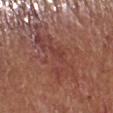Case summary:
* notes: no biopsy performed (imaged during a skin exam)
* image: ~15 mm crop, total-body skin-cancer survey
* site: the leg
* lighting: white-light illumination
* image-analysis metrics: a classifier nevus-likeness of about 0/100
* subject: male, aged 73 to 77